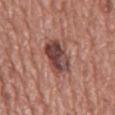imaging modality = ~15 mm crop, total-body skin-cancer survey | anatomic site = the chest | patient = male, aged approximately 75.